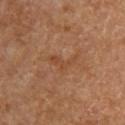Q: Was a biopsy performed?
A: total-body-photography surveillance lesion; no biopsy
Q: What is the lesion's diameter?
A: ~4 mm (longest diameter)
Q: Where on the body is the lesion?
A: the back
Q: Automated lesion metrics?
A: an area of roughly 5.5 mm², an outline eccentricity of about 0.8 (0 = round, 1 = elongated), and a shape-asymmetry score of about 0.7 (0 = symmetric); a mean CIELAB color near L≈42 a*≈20 b*≈31 and a normalized lesion–skin contrast near 5; border irregularity of about 8 on a 0–10 scale
Q: What is the imaging modality?
A: ~15 mm crop, total-body skin-cancer survey
Q: What are the patient's age and sex?
A: female, aged 58–62
Q: What lighting was used for the tile?
A: cross-polarized illumination